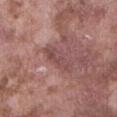No biopsy was performed on this lesion — it was imaged during a full skin examination and was not determined to be concerning.
Approximately 4.5 mm at its widest.
The subject is a male approximately 75 years of age.
This is a white-light tile.
A 15 mm crop from a total-body photograph taken for skin-cancer surveillance.
Located on the abdomen.